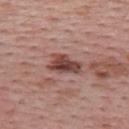Recorded during total-body skin imaging; not selected for excision or biopsy. Imaged with white-light lighting. A female patient, roughly 55 years of age. A 15 mm crop from a total-body photograph taken for skin-cancer surveillance. The lesion is located on the upper back.